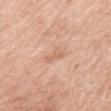Impression:
Recorded during total-body skin imaging; not selected for excision or biopsy.
Image and clinical context:
Measured at roughly 3 mm in maximum diameter. A 15 mm close-up tile from a total-body photography series done for melanoma screening. Imaged with white-light lighting. The lesion is located on the mid back. A female subject, aged around 70.